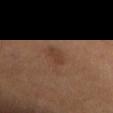The lesion was tiled from a total-body skin photograph and was not biopsied. A region of skin cropped from a whole-body photographic capture, roughly 15 mm wide. The subject is a male aged approximately 65. Automated image analysis of the tile measured a lesion area of about 3.5 mm², an eccentricity of roughly 0.8, and a symmetry-axis asymmetry near 0.15. The software also gave an average lesion color of about L≈38 a*≈19 b*≈28 (CIELAB). The lesion's longest dimension is about 2.5 mm. This is a cross-polarized tile.